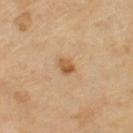Notes:
• subject · female, roughly 70 years of age
• diameter · about 2 mm
• site · the left thigh
• image · ~15 mm crop, total-body skin-cancer survey
• image-analysis metrics · a lesion area of about 3 mm², an outline eccentricity of about 0.55 (0 = round, 1 = elongated), and two-axis asymmetry of about 0.25; an average lesion color of about L≈57 a*≈20 b*≈39 (CIELAB) and a normalized border contrast of about 8; a nevus-likeness score of about 85/100 and lesion-presence confidence of about 100/100
• illumination · cross-polarized illumination